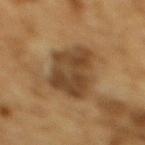Imaged during a routine full-body skin examination; the lesion was not biopsied and no histopathology is available. A male patient, roughly 85 years of age or older. The lesion is on the mid back. This image is a 15 mm lesion crop taken from a total-body photograph.